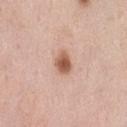* notes: catalogued during a skin exam; not biopsied
* acquisition: 15 mm crop, total-body photography
* tile lighting: white-light illumination
* diameter: ~3 mm (longest diameter)
* patient: female, about 50 years old
* anatomic site: the left thigh
* automated lesion analysis: a within-lesion color-variation index near 4/10 and peripheral color asymmetry of about 1; an automated nevus-likeness rating near 100 out of 100